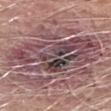No biopsy was performed on this lesion — it was imaged during a full skin examination and was not determined to be concerning. From the right forearm. The tile uses white-light illumination. The patient is a male about 65 years old. Cropped from a total-body skin-imaging series; the visible field is about 15 mm.